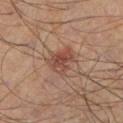Impression: Captured during whole-body skin photography for melanoma surveillance; the lesion was not biopsied. Clinical summary: A region of skin cropped from a whole-body photographic capture, roughly 15 mm wide. The patient is a male aged around 55. Longest diameter approximately 3.5 mm. The lesion is on the leg. Automated tile analysis of the lesion measured a shape eccentricity near 0.7 and a symmetry-axis asymmetry near 0.3. The software also gave a border-irregularity rating of about 3.5/10, a within-lesion color-variation index near 4/10, and radial color variation of about 1. Imaged with cross-polarized lighting.